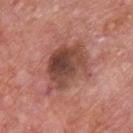– biopsy status — imaged on a skin check; not biopsied
– anatomic site — the chest
– subject — male, aged approximately 75
– lesion diameter — ≈6 mm
– imaging modality — ~15 mm tile from a whole-body skin photo